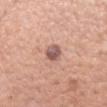Impression:
This lesion was catalogued during total-body skin photography and was not selected for biopsy.
Acquisition and patient details:
This is a white-light tile. A female subject approximately 60 years of age. Cropped from a total-body skin-imaging series; the visible field is about 15 mm. About 2.5 mm across. Located on the left forearm.